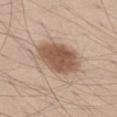– lighting — white-light illumination
– anatomic site — the left thigh
– subject — male, in their 40s
– image — 15 mm crop, total-body photography
– lesion size — ~6 mm (longest diameter)
– histopathology — a melanoma in situ (malignant)